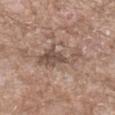{
  "patient": {
    "sex": "male",
    "age_approx": 50
  },
  "site": "left forearm",
  "lighting": "white-light",
  "lesion_size": {
    "long_diameter_mm_approx": 5.5
  },
  "image": {
    "source": "total-body photography crop",
    "field_of_view_mm": 15
  }
}